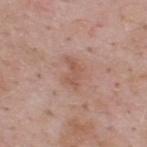The lesion is located on the upper back.
Imaged with white-light lighting.
The total-body-photography lesion software estimated a lesion color around L≈54 a*≈21 b*≈27 in CIELAB, a lesion–skin lightness drop of about 8, and a lesion-to-skin contrast of about 6 (normalized; higher = more distinct). The analysis additionally found a nevus-likeness score of about 5/100.
A 15 mm crop from a total-body photograph taken for skin-cancer surveillance.
A male patient aged 53 to 57.